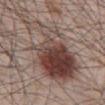{"patient": {"sex": "male", "age_approx": 65}, "image": {"source": "total-body photography crop", "field_of_view_mm": 15}, "lighting": "white-light", "lesion_size": {"long_diameter_mm_approx": 12.0}, "automated_metrics": {"eccentricity": 0.8, "shape_asymmetry": 0.35, "cielab_L": 44, "cielab_a": 17, "cielab_b": 21, "vs_skin_darker_L": 13.0, "vs_skin_contrast_norm": 10.0}, "site": "front of the torso"}